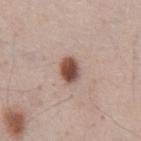The lesion was tiled from a total-body skin photograph and was not biopsied. Captured under white-light illumination. The recorded lesion diameter is about 3 mm. A 15 mm crop from a total-body photograph taken for skin-cancer surveillance. The patient is a male in their mid-50s. The lesion is on the abdomen.